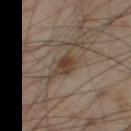Q: What is the imaging modality?
A: ~15 mm crop, total-body skin-cancer survey
Q: Who is the patient?
A: male, aged 53 to 57
Q: Lesion size?
A: ~2.5 mm (longest diameter)
Q: What did automated image analysis measure?
A: a lesion area of about 4.5 mm², an eccentricity of roughly 0.45, and a shape-asymmetry score of about 0.3 (0 = symmetric); an average lesion color of about L≈39 a*≈14 b*≈25 (CIELAB), about 9 CIELAB-L* units darker than the surrounding skin, and a normalized border contrast of about 8; a border-irregularity index near 2.5/10, a within-lesion color-variation index near 4.5/10, and a peripheral color-asymmetry measure near 1.5
Q: Where on the body is the lesion?
A: the leg
Q: How was the tile lit?
A: cross-polarized illumination